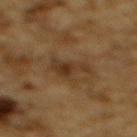Q: Is there a histopathology result?
A: no biopsy performed (imaged during a skin exam)
Q: How was this image acquired?
A: ~15 mm crop, total-body skin-cancer survey
Q: What are the patient's age and sex?
A: male, aged around 85 or older
Q: Lesion location?
A: the back